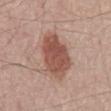Impression:
Part of a total-body skin-imaging series; this lesion was reviewed on a skin check and was not flagged for biopsy.
Background:
A region of skin cropped from a whole-body photographic capture, roughly 15 mm wide. Measured at roughly 7 mm in maximum diameter. The lesion-visualizer software estimated a footprint of about 17 mm² and an eccentricity of roughly 0.9. And it measured an average lesion color of about L≈51 a*≈23 b*≈26 (CIELAB), about 13 CIELAB-L* units darker than the surrounding skin, and a normalized lesion–skin contrast near 9. On the mid back. A male subject aged approximately 70. Captured under white-light illumination.